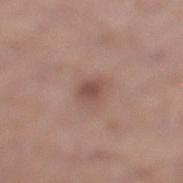The lesion was tiled from a total-body skin photograph and was not biopsied. A lesion tile, about 15 mm wide, cut from a 3D total-body photograph. An algorithmic analysis of the crop reported a border-irregularity rating of about 2/10 and a within-lesion color-variation index near 2.5/10. It also reported a detector confidence of about 100 out of 100 that the crop contains a lesion. A male patient, aged approximately 55. From the left lower leg. Imaged with white-light lighting. Measured at roughly 2.5 mm in maximum diameter.